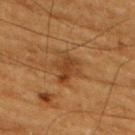Clinical impression: The lesion was photographed on a routine skin check and not biopsied; there is no pathology result.